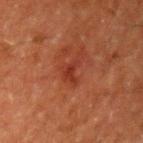Q: Is there a histopathology result?
A: catalogued during a skin exam; not biopsied
Q: How was this image acquired?
A: ~15 mm tile from a whole-body skin photo
Q: What is the anatomic site?
A: the left upper arm
Q: Lesion size?
A: ≈3 mm
Q: What lighting was used for the tile?
A: cross-polarized illumination
Q: What are the patient's age and sex?
A: male, aged 58–62